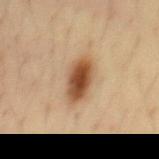- biopsy status · catalogued during a skin exam; not biopsied
- lesion diameter · about 5 mm
- lighting · cross-polarized
- site · the front of the torso
- imaging modality · ~15 mm crop, total-body skin-cancer survey
- subject · male, aged approximately 35